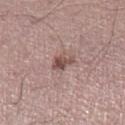lighting: white-light illumination; location: the left lower leg; imaging modality: 15 mm crop, total-body photography; patient: male, aged 58–62.